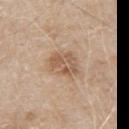Captured during whole-body skin photography for melanoma surveillance; the lesion was not biopsied. Cropped from a total-body skin-imaging series; the visible field is about 15 mm. The recorded lesion diameter is about 4 mm. A male patient aged 78–82. The lesion is on the arm.